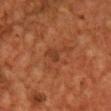Notes:
• follow-up · no biopsy performed (imaged during a skin exam)
• subject · male, about 60 years old
• automated metrics · a footprint of about 7 mm², an outline eccentricity of about 0.8 (0 = round, 1 = elongated), and a shape-asymmetry score of about 0.65 (0 = symmetric); border irregularity of about 7 on a 0–10 scale and a within-lesion color-variation index near 2/10
• anatomic site · the chest
• imaging modality · total-body-photography crop, ~15 mm field of view
• tile lighting · cross-polarized